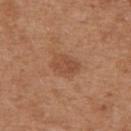The recorded lesion diameter is about 3.5 mm.
Located on the back.
This is a white-light tile.
A 15 mm close-up extracted from a 3D total-body photography capture.
A female subject, aged around 40.